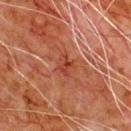Clinical impression:
Imaged during a routine full-body skin examination; the lesion was not biopsied and no histopathology is available.
Acquisition and patient details:
A male patient, roughly 80 years of age. The lesion is located on the chest. A close-up tile cropped from a whole-body skin photograph, about 15 mm across.